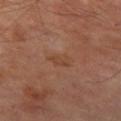Recorded during total-body skin imaging; not selected for excision or biopsy.
A 15 mm crop from a total-body photograph taken for skin-cancer surveillance.
Captured under cross-polarized illumination.
Located on the left thigh.
Automated tile analysis of the lesion measured a footprint of about 4 mm², a shape eccentricity near 0.85, and a symmetry-axis asymmetry near 0.3. And it measured an average lesion color of about L≈42 a*≈21 b*≈29 (CIELAB), roughly 5 lightness units darker than nearby skin, and a normalized lesion–skin contrast near 4.5.
Measured at roughly 3 mm in maximum diameter.
A male subject, approximately 70 years of age.